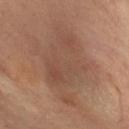Assessment: Recorded during total-body skin imaging; not selected for excision or biopsy. Background: The recorded lesion diameter is about 9.5 mm. Imaged with cross-polarized lighting. A female subject, aged 68–72. A 15 mm close-up extracted from a 3D total-body photography capture. The lesion is located on the right lower leg.